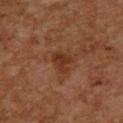• biopsy status — total-body-photography surveillance lesion; no biopsy
• site — the upper back
• image source — ~15 mm crop, total-body skin-cancer survey
• patient — female, aged around 60